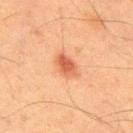The lesion was photographed on a routine skin check and not biopsied; there is no pathology result. Imaged with cross-polarized lighting. On the mid back. Automated image analysis of the tile measured a mean CIELAB color near L≈48 a*≈24 b*≈32 and a normalized border contrast of about 7.5. The software also gave a border-irregularity index near 2/10 and radial color variation of about 1. The software also gave a classifier nevus-likeness of about 95/100 and a detector confidence of about 100 out of 100 that the crop contains a lesion. The patient is a male about 35 years old. This image is a 15 mm lesion crop taken from a total-body photograph. The recorded lesion diameter is about 3.5 mm.